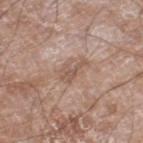notes=total-body-photography surveillance lesion; no biopsy
site=the leg
size=~3.5 mm (longest diameter)
imaging modality=~15 mm tile from a whole-body skin photo
subject=male, aged 58–62